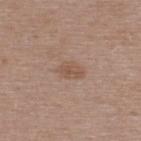- body site · the upper back
- patient · female, in their mid-40s
- image source · ~15 mm crop, total-body skin-cancer survey